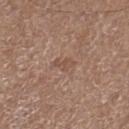A 15 mm close-up tile from a total-body photography series done for melanoma screening.
A male subject in their mid- to late 70s.
Approximately 2.5 mm at its widest.
The lesion is on the right lower leg.
The tile uses white-light illumination.